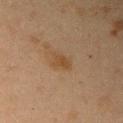Q: Is there a histopathology result?
A: total-body-photography surveillance lesion; no biopsy
Q: How large is the lesion?
A: about 2.5 mm
Q: What kind of image is this?
A: total-body-photography crop, ~15 mm field of view
Q: What is the anatomic site?
A: the right upper arm
Q: What are the patient's age and sex?
A: female, aged 38–42
Q: Automated lesion metrics?
A: a shape eccentricity near 0.75 and a symmetry-axis asymmetry near 0.35; a lesion color around L≈38 a*≈15 b*≈30 in CIELAB, a lesion–skin lightness drop of about 6, and a lesion-to-skin contrast of about 7 (normalized; higher = more distinct); a border-irregularity index near 4/10, internal color variation of about 1.5 on a 0–10 scale, and radial color variation of about 0.5; a classifier nevus-likeness of about 15/100 and a detector confidence of about 100 out of 100 that the crop contains a lesion
Q: What lighting was used for the tile?
A: cross-polarized illumination